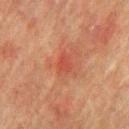Clinical summary:
From the chest. An algorithmic analysis of the crop reported an average lesion color of about L≈42 a*≈29 b*≈30 (CIELAB) and a lesion–skin lightness drop of about 6. It also reported border irregularity of about 3.5 on a 0–10 scale and a peripheral color-asymmetry measure near 1. And it measured a nevus-likeness score of about 0/100 and lesion-presence confidence of about 100/100. A male subject roughly 75 years of age. Captured under cross-polarized illumination. The recorded lesion diameter is about 3 mm. A region of skin cropped from a whole-body photographic capture, roughly 15 mm wide.